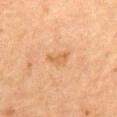Q: What did automated image analysis measure?
A: a shape eccentricity near 0.85 and two-axis asymmetry of about 0.5; a border-irregularity index near 4.5/10 and internal color variation of about 1 on a 0–10 scale; a nevus-likeness score of about 0/100 and lesion-presence confidence of about 100/100
Q: What is the imaging modality?
A: ~15 mm crop, total-body skin-cancer survey
Q: How was the tile lit?
A: cross-polarized illumination
Q: Patient demographics?
A: female, aged approximately 60
Q: Where on the body is the lesion?
A: the abdomen
Q: Lesion size?
A: ≈3 mm